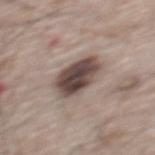Clinical impression: Part of a total-body skin-imaging series; this lesion was reviewed on a skin check and was not flagged for biopsy. Background: Automated image analysis of the tile measured a footprint of about 14 mm², an outline eccentricity of about 0.65 (0 = round, 1 = elongated), and two-axis asymmetry of about 0.15. It also reported a classifier nevus-likeness of about 35/100. Cropped from a total-body skin-imaging series; the visible field is about 15 mm. A male subject, roughly 65 years of age. Captured under white-light illumination. Approximately 4.5 mm at its widest. The lesion is located on the upper back.